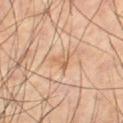Case summary:
- workup · total-body-photography surveillance lesion; no biopsy
- subject · male, approximately 60 years of age
- lighting · cross-polarized illumination
- image · ~15 mm tile from a whole-body skin photo
- site · the left thigh